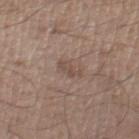The lesion was tiled from a total-body skin photograph and was not biopsied. Captured under white-light illumination. The lesion's longest dimension is about 3 mm. A male subject, in their mid-50s. The lesion is located on the right thigh. Automated tile analysis of the lesion measured a mean CIELAB color near L≈49 a*≈16 b*≈24, a lesion–skin lightness drop of about 7, and a lesion-to-skin contrast of about 5.5 (normalized; higher = more distinct). And it measured a color-variation rating of about 0.5/10 and radial color variation of about 0. It also reported a nevus-likeness score of about 0/100. A 15 mm close-up extracted from a 3D total-body photography capture.